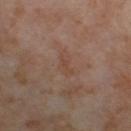About 3 mm across.
This image is a 15 mm lesion crop taken from a total-body photograph.
A female subject approximately 55 years of age.
The lesion is located on the right thigh.